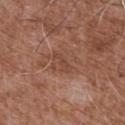Assessment: Part of a total-body skin-imaging series; this lesion was reviewed on a skin check and was not flagged for biopsy. Context: Longest diameter approximately 3 mm. A 15 mm crop from a total-body photograph taken for skin-cancer surveillance. A male subject, aged around 75. Captured under white-light illumination. The lesion is located on the front of the torso.